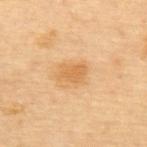Findings:
* biopsy status — imaged on a skin check; not biopsied
* subject — female, aged approximately 65
* image — total-body-photography crop, ~15 mm field of view
* image-analysis metrics — a lesion area of about 5 mm², a shape eccentricity near 0.7, and a shape-asymmetry score of about 0.25 (0 = symmetric); a border-irregularity index near 2.5/10 and a within-lesion color-variation index near 2/10
* body site — the upper back
* lesion size — ~3 mm (longest diameter)